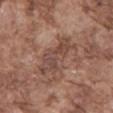Assessment:
The lesion was photographed on a routine skin check and not biopsied; there is no pathology result.
Context:
Cropped from a whole-body photographic skin survey; the tile spans about 15 mm. A male patient in their mid- to late 70s. From the mid back.